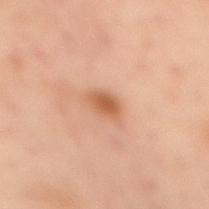The lesion was tiled from a total-body skin photograph and was not biopsied. Cropped from a whole-body photographic skin survey; the tile spans about 15 mm. Automated image analysis of the tile measured an average lesion color of about L≈49 a*≈21 b*≈30 (CIELAB) and a normalized border contrast of about 7.5. The subject is a female approximately 55 years of age. Located on the mid back. About 2.5 mm across. Imaged with cross-polarized lighting.